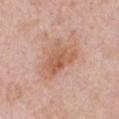The lesion was photographed on a routine skin check and not biopsied; there is no pathology result.
A region of skin cropped from a whole-body photographic capture, roughly 15 mm wide.
The lesion is on the chest.
A female subject, in their 50s.